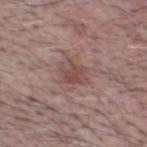Impression:
The lesion was photographed on a routine skin check and not biopsied; there is no pathology result.
Acquisition and patient details:
This is a white-light tile. This image is a 15 mm lesion crop taken from a total-body photograph. The lesion is located on the head or neck. A male patient, aged 58 to 62. An algorithmic analysis of the crop reported a lesion color around L≈47 a*≈21 b*≈21 in CIELAB and about 8 CIELAB-L* units darker than the surrounding skin. The software also gave lesion-presence confidence of about 100/100.